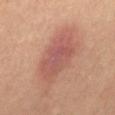workup: imaged on a skin check; not biopsied | subject: male, about 70 years old | imaging modality: ~15 mm tile from a whole-body skin photo | body site: the mid back | diameter: about 6.5 mm | lighting: cross-polarized.